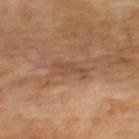The lesion was tiled from a total-body skin photograph and was not biopsied.
The lesion is on the upper back.
The lesion's longest dimension is about 4 mm.
Captured under cross-polarized illumination.
The total-body-photography lesion software estimated an area of roughly 5 mm², a shape eccentricity near 0.95, and a symmetry-axis asymmetry near 0.35. The software also gave a mean CIELAB color near L≈50 a*≈22 b*≈32, roughly 8 lightness units darker than nearby skin, and a normalized border contrast of about 5.5. And it measured a border-irregularity rating of about 4.5/10, a color-variation rating of about 1/10, and a peripheral color-asymmetry measure near 0.5. The software also gave a nevus-likeness score of about 0/100 and a detector confidence of about 95 out of 100 that the crop contains a lesion.
A region of skin cropped from a whole-body photographic capture, roughly 15 mm wide.